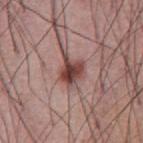This lesion was catalogued during total-body skin photography and was not selected for biopsy. About 4 mm across. Located on the abdomen. Captured under white-light illumination. A region of skin cropped from a whole-body photographic capture, roughly 15 mm wide. A male patient, roughly 55 years of age.